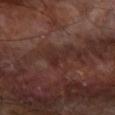The lesion was tiled from a total-body skin photograph and was not biopsied. A male subject about 65 years old. Cropped from a total-body skin-imaging series; the visible field is about 15 mm. Captured under cross-polarized illumination. Located on the right forearm. Automated image analysis of the tile measured a lesion area of about 4 mm², an eccentricity of roughly 0.8, and a shape-asymmetry score of about 0.6 (0 = symmetric). The analysis additionally found a mean CIELAB color near L≈26 a*≈19 b*≈20 and roughly 5 lightness units darker than nearby skin. And it measured border irregularity of about 6.5 on a 0–10 scale, internal color variation of about 1 on a 0–10 scale, and radial color variation of about 0.5.